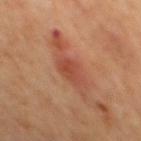Clinical impression:
Part of a total-body skin-imaging series; this lesion was reviewed on a skin check and was not flagged for biopsy.
Background:
Measured at roughly 3.5 mm in maximum diameter. A 15 mm crop from a total-body photograph taken for skin-cancer surveillance. Captured under cross-polarized illumination. The lesion is located on the mid back. The patient is a male roughly 65 years of age.